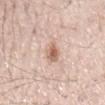| field | value |
|---|---|
| biopsy status | catalogued during a skin exam; not biopsied |
| location | the mid back |
| image | ~15 mm crop, total-body skin-cancer survey |
| patient | male, approximately 80 years of age |